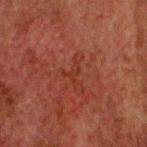Recorded during total-body skin imaging; not selected for excision or biopsy. Located on the head or neck. Automated tile analysis of the lesion measured a classifier nevus-likeness of about 0/100 and a lesion-detection confidence of about 85/100. A lesion tile, about 15 mm wide, cut from a 3D total-body photograph. A male patient, aged around 60. About 2.5 mm across.